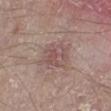follow-up = no biopsy performed (imaged during a skin exam) | image-analysis metrics = an average lesion color of about L≈51 a*≈19 b*≈20 (CIELAB) and a normalized border contrast of about 5; a border-irregularity rating of about 6/10, a color-variation rating of about 4/10, and a peripheral color-asymmetry measure near 1.5; a classifier nevus-likeness of about 0/100 and a lesion-detection confidence of about 100/100 | image = total-body-photography crop, ~15 mm field of view | tile lighting = white-light | anatomic site = the left lower leg | subject = male, in their mid-60s | lesion diameter = about 4 mm.